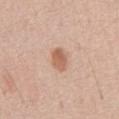notes = total-body-photography surveillance lesion; no biopsy
subject = male, roughly 70 years of age
anatomic site = the abdomen
acquisition = total-body-photography crop, ~15 mm field of view